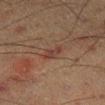notes — total-body-photography surveillance lesion; no biopsy
location — the right lower leg
subject — male, roughly 60 years of age
image — 15 mm crop, total-body photography
lesion diameter — ~3 mm (longest diameter)
lighting — cross-polarized illumination